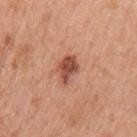Q: Was a biopsy performed?
A: no biopsy performed (imaged during a skin exam)
Q: What kind of image is this?
A: 15 mm crop, total-body photography
Q: Lesion location?
A: the arm
Q: Who is the patient?
A: female, in their 40s
Q: What lighting was used for the tile?
A: white-light
Q: How large is the lesion?
A: ~3.5 mm (longest diameter)
Q: Automated lesion metrics?
A: an average lesion color of about L≈50 a*≈27 b*≈31 (CIELAB), a lesion–skin lightness drop of about 14, and a normalized border contrast of about 9.5